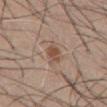Case summary:
– site: the abdomen
– subject: male, approximately 55 years of age
– tile lighting: white-light
– automated lesion analysis: border irregularity of about 3 on a 0–10 scale, internal color variation of about 4 on a 0–10 scale, and peripheral color asymmetry of about 1
– lesion size: about 3 mm
– acquisition: 15 mm crop, total-body photography